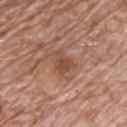imaging modality — total-body-photography crop, ~15 mm field of view; site — the chest; patient — male, aged around 70.